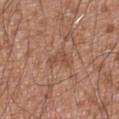The lesion was photographed on a routine skin check and not biopsied; there is no pathology result. A male subject in their mid-70s. From the abdomen. A close-up tile cropped from a whole-body skin photograph, about 15 mm across.